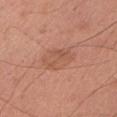biopsy status — total-body-photography surveillance lesion; no biopsy
body site — the left upper arm
subject — male, about 60 years old
illumination — white-light illumination
acquisition — total-body-photography crop, ~15 mm field of view
diameter — ~4 mm (longest diameter)
automated lesion analysis — a lesion area of about 9.5 mm² and an outline eccentricity of about 0.65 (0 = round, 1 = elongated); an average lesion color of about L≈55 a*≈24 b*≈31 (CIELAB), roughly 7 lightness units darker than nearby skin, and a normalized lesion–skin contrast near 4.5; a border-irregularity index near 2/10, internal color variation of about 3 on a 0–10 scale, and a peripheral color-asymmetry measure near 1